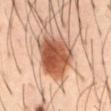<lesion>
<biopsy_status>not biopsied; imaged during a skin examination</biopsy_status>
<patient>
  <sex>male</sex>
  <age_approx>40</age_approx>
</patient>
<lighting>cross-polarized</lighting>
<image>
  <source>total-body photography crop</source>
  <field_of_view_mm>15</field_of_view_mm>
</image>
<site>abdomen</site>
<automated_metrics>
  <border_irregularity_0_10>2.5</border_irregularity_0_10>
  <color_variation_0_10>7.0</color_variation_0_10>
  <peripheral_color_asymmetry>2.0</peripheral_color_asymmetry>
</automated_metrics>
<lesion_size>
  <long_diameter_mm_approx>6.0</long_diameter_mm_approx>
</lesion_size>
</lesion>